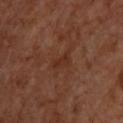workup: catalogued during a skin exam; not biopsied | tile lighting: cross-polarized | body site: the back | patient: male, about 70 years old | size: ~3 mm (longest diameter) | TBP lesion metrics: an eccentricity of roughly 0.85 and a shape-asymmetry score of about 0.4 (0 = symmetric); a mean CIELAB color near L≈29 a*≈22 b*≈27, about 5 CIELAB-L* units darker than the surrounding skin, and a lesion-to-skin contrast of about 5.5 (normalized; higher = more distinct); an automated nevus-likeness rating near 0 out of 100 and a lesion-detection confidence of about 100/100 | image source: total-body-photography crop, ~15 mm field of view.